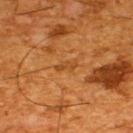Findings:
* workup · no biopsy performed (imaged during a skin exam)
* lighting · cross-polarized illumination
* acquisition · 15 mm crop, total-body photography
* subject · male, aged around 65
* size · ~2.5 mm (longest diameter)
* TBP lesion metrics · a lesion area of about 3 mm², a shape eccentricity near 0.9, and a shape-asymmetry score of about 0.25 (0 = symmetric); a mean CIELAB color near L≈38 a*≈22 b*≈37, a lesion–skin lightness drop of about 6, and a lesion-to-skin contrast of about 5 (normalized; higher = more distinct); a border-irregularity index near 3/10; a classifier nevus-likeness of about 0/100
* anatomic site · the upper back